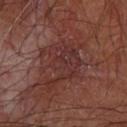Recorded during total-body skin imaging; not selected for excision or biopsy.
A male patient, aged 63 to 67.
The recorded lesion diameter is about 7 mm.
Located on the left forearm.
A 15 mm close-up extracted from a 3D total-body photography capture.
Imaged with cross-polarized lighting.